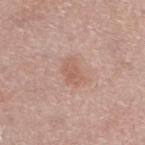This lesion was catalogued during total-body skin photography and was not selected for biopsy.
From the leg.
The patient is a male aged around 60.
A 15 mm close-up extracted from a 3D total-body photography capture.